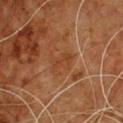No biopsy was performed on this lesion — it was imaged during a full skin examination and was not determined to be concerning. The lesion is located on the chest. The subject is a male roughly 60 years of age. Captured under cross-polarized illumination. Cropped from a total-body skin-imaging series; the visible field is about 15 mm. Approximately 3 mm at its widest.